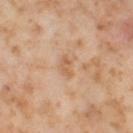Q: Was a biopsy performed?
A: catalogued during a skin exam; not biopsied
Q: How large is the lesion?
A: ~2.5 mm (longest diameter)
Q: What is the anatomic site?
A: the left thigh
Q: Patient demographics?
A: female, aged 53 to 57
Q: What is the imaging modality?
A: ~15 mm tile from a whole-body skin photo
Q: What did automated image analysis measure?
A: a footprint of about 2.5 mm² and a shape eccentricity near 0.9; roughly 8 lightness units darker than nearby skin and a normalized border contrast of about 5.5; a nevus-likeness score of about 0/100
Q: Illumination type?
A: cross-polarized illumination